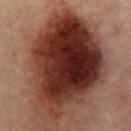  image:
    source: total-body photography crop
    field_of_view_mm: 15
  automated_metrics:
    cielab_L: 27
    cielab_a: 20
    cielab_b: 22
    vs_skin_darker_L: 18.0
    nevus_likeness_0_100: 100
    lesion_detection_confidence_0_100: 100
  lesion_size:
    long_diameter_mm_approx: 14.0
  patient:
    sex: female
    age_approx: 55
  lighting: cross-polarized
  site: abdomen